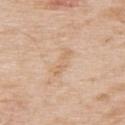notes: no biopsy performed (imaged during a skin exam); body site: the upper back; tile lighting: white-light illumination; imaging modality: 15 mm crop, total-body photography; subject: male, roughly 65 years of age.